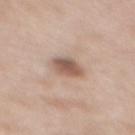Automated image analysis of the tile measured a footprint of about 6 mm² and a shape-asymmetry score of about 0.2 (0 = symmetric).
The tile uses white-light illumination.
The subject is a female aged 38 to 42.
On the mid back.
A region of skin cropped from a whole-body photographic capture, roughly 15 mm wide.